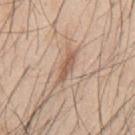<record>
  <biopsy_status>not biopsied; imaged during a skin examination</biopsy_status>
  <patient>
    <sex>male</sex>
    <age_approx>45</age_approx>
  </patient>
  <site>back</site>
  <automated_metrics>
    <area_mm2_approx>4.0</area_mm2_approx>
    <eccentricity>0.95</eccentricity>
    <shape_asymmetry>0.2</shape_asymmetry>
  </automated_metrics>
  <image>
    <source>total-body photography crop</source>
    <field_of_view_mm>15</field_of_view_mm>
  </image>
</record>